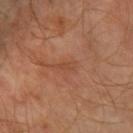follow-up: catalogued during a skin exam; not biopsied | tile lighting: cross-polarized illumination | automated metrics: an area of roughly 2.5 mm² and a shape-asymmetry score of about 0.4 (0 = symmetric); a border-irregularity index near 4/10 and radial color variation of about 0 | size: ≈2.5 mm | image source: ~15 mm tile from a whole-body skin photo | subject: male, roughly 60 years of age | body site: the right forearm.